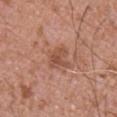Q: Was this lesion biopsied?
A: total-body-photography surveillance lesion; no biopsy
Q: Where on the body is the lesion?
A: the abdomen
Q: Patient demographics?
A: male, aged 73 to 77
Q: What lighting was used for the tile?
A: white-light illumination
Q: How was this image acquired?
A: ~15 mm tile from a whole-body skin photo
Q: What did automated image analysis measure?
A: about 8 CIELAB-L* units darker than the surrounding skin and a normalized lesion–skin contrast near 6